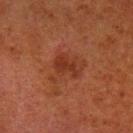| field | value |
|---|---|
| notes | imaged on a skin check; not biopsied |
| lesion diameter | ~3 mm (longest diameter) |
| automated metrics | a lesion–skin lightness drop of about 6; a classifier nevus-likeness of about 0/100 |
| location | the left lower leg |
| image source | total-body-photography crop, ~15 mm field of view |
| lighting | cross-polarized |
| patient | male, aged around 80 |